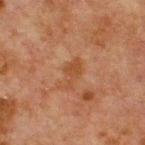  biopsy_status: not biopsied; imaged during a skin examination